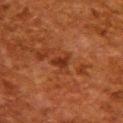No biopsy was performed on this lesion — it was imaged during a full skin examination and was not determined to be concerning.
The total-body-photography lesion software estimated a mean CIELAB color near L≈28 a*≈24 b*≈30 and a lesion-to-skin contrast of about 7.5 (normalized; higher = more distinct). The analysis additionally found a border-irregularity index near 4/10 and a color-variation rating of about 1/10.
From the upper back.
Cropped from a whole-body photographic skin survey; the tile spans about 15 mm.
This is a cross-polarized tile.
A female patient about 50 years old.